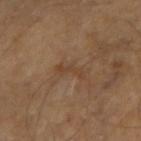This lesion was catalogued during total-body skin photography and was not selected for biopsy. The lesion is on the right forearm. Captured under cross-polarized illumination. About 3.5 mm across. Cropped from a whole-body photographic skin survey; the tile spans about 15 mm. The patient is a male aged 68 to 72.